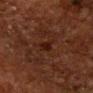This lesion was catalogued during total-body skin photography and was not selected for biopsy.